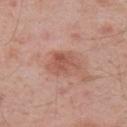Findings:
* biopsy status · catalogued during a skin exam; not biopsied
* imaging modality · total-body-photography crop, ~15 mm field of view
* patient · male, in their mid- to late 50s
* anatomic site · the right upper arm